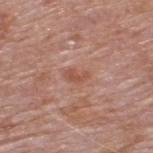Impression: This lesion was catalogued during total-body skin photography and was not selected for biopsy. Image and clinical context: Located on the upper back. The lesion's longest dimension is about 3 mm. This image is a 15 mm lesion crop taken from a total-body photograph. Captured under white-light illumination. A male patient roughly 60 years of age. Automated image analysis of the tile measured a shape eccentricity near 0.85 and two-axis asymmetry of about 0.4. The software also gave a lesion color around L≈52 a*≈25 b*≈29 in CIELAB, about 8 CIELAB-L* units darker than the surrounding skin, and a normalized lesion–skin contrast near 6. The software also gave a classifier nevus-likeness of about 0/100 and lesion-presence confidence of about 100/100.